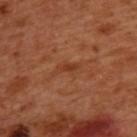  biopsy_status: not biopsied; imaged during a skin examination
  automated_metrics:
    border_irregularity_0_10: 4.0
    color_variation_0_10: 0.0
    peripheral_color_asymmetry: 0.0
  site: back
  patient:
    sex: male
    age_approx: 50
  image:
    source: total-body photography crop
    field_of_view_mm: 15
  lesion_size:
    long_diameter_mm_approx: 2.5
  lighting: cross-polarized